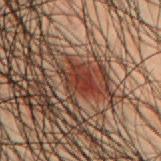follow-up = no biopsy performed (imaged during a skin exam) | imaging modality = ~15 mm tile from a whole-body skin photo | lesion diameter = ≈3.5 mm | location = the mid back | subject = male, aged around 50 | TBP lesion metrics = an area of roughly 7.5 mm² and two-axis asymmetry of about 0.3; a border-irregularity index near 3/10, a color-variation rating of about 5/10, and radial color variation of about 2 | illumination = cross-polarized illumination.